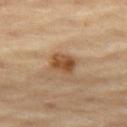Impression:
This lesion was catalogued during total-body skin photography and was not selected for biopsy.
Acquisition and patient details:
A lesion tile, about 15 mm wide, cut from a 3D total-body photograph. The lesion is on the left thigh. A female patient, aged 68–72. Approximately 3 mm at its widest. Captured under cross-polarized illumination.